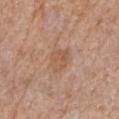Recorded during total-body skin imaging; not selected for excision or biopsy. About 3.5 mm across. This is a white-light tile. The total-body-photography lesion software estimated an area of roughly 6.5 mm², an eccentricity of roughly 0.7, and two-axis asymmetry of about 0.25. The analysis additionally found an average lesion color of about L≈55 a*≈20 b*≈31 (CIELAB), about 7 CIELAB-L* units darker than the surrounding skin, and a normalized lesion–skin contrast near 5.5. The analysis additionally found a border-irregularity rating of about 2.5/10, a color-variation rating of about 2.5/10, and a peripheral color-asymmetry measure near 1. And it measured a classifier nevus-likeness of about 0/100. On the right forearm. A female patient in their 60s. A 15 mm close-up extracted from a 3D total-body photography capture.